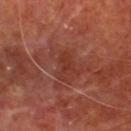Part of a total-body skin-imaging series; this lesion was reviewed on a skin check and was not flagged for biopsy. The recorded lesion diameter is about 3.5 mm. On the chest. The tile uses cross-polarized illumination. A male patient, aged approximately 65. Automated tile analysis of the lesion measured an eccentricity of roughly 0.9 and two-axis asymmetry of about 0.45. The analysis additionally found a mean CIELAB color near L≈34 a*≈26 b*≈28, roughly 6 lightness units darker than nearby skin, and a normalized border contrast of about 6. A 15 mm crop from a total-body photograph taken for skin-cancer surveillance.